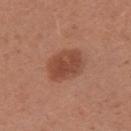Case summary:
* illumination · white-light
* lesion diameter · ≈4 mm
* site · the right upper arm
* image · 15 mm crop, total-body photography
* TBP lesion metrics · a lesion color around L≈46 a*≈24 b*≈30 in CIELAB, a lesion–skin lightness drop of about 10, and a normalized lesion–skin contrast near 7.5
* patient · female, aged approximately 25